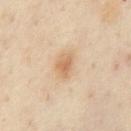Background: A 15 mm close-up tile from a total-body photography series done for melanoma screening. Located on the chest. A male patient, in their mid-50s. Longest diameter approximately 2.5 mm.